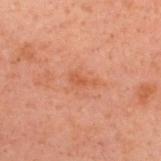<tbp_lesion>
  <biopsy_status>not biopsied; imaged during a skin examination</biopsy_status>
  <patient>
    <sex>male</sex>
    <age_approx>40</age_approx>
  </patient>
  <automated_metrics>
    <area_mm2_approx>2.5</area_mm2_approx>
    <eccentricity>0.85</eccentricity>
    <shape_asymmetry>0.45</shape_asymmetry>
    <cielab_L>45</cielab_L>
    <cielab_a>24</cielab_a>
    <cielab_b>31</cielab_b>
    <vs_skin_darker_L>6.0</vs_skin_darker_L>
    <border_irregularity_0_10>5.0</border_irregularity_0_10>
    <color_variation_0_10>0.5</color_variation_0_10>
    <peripheral_color_asymmetry>0.0</peripheral_color_asymmetry>
    <nevus_likeness_0_100>0</nevus_likeness_0_100>
  </automated_metrics>
  <site>upper back</site>
  <lesion_size>
    <long_diameter_mm_approx>2.5</long_diameter_mm_approx>
  </lesion_size>
  <image>
    <source>total-body photography crop</source>
    <field_of_view_mm>15</field_of_view_mm>
  </image>
  <lighting>cross-polarized</lighting>
</tbp_lesion>